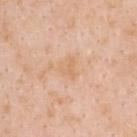workup: no biopsy performed (imaged during a skin exam); body site: the upper back; lesion size: ≈2.5 mm; patient: female, in their 40s; acquisition: 15 mm crop, total-body photography; lighting: white-light.